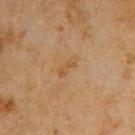biopsy status: no biopsy performed (imaged during a skin exam) | anatomic site: the back | lesion diameter: ~3 mm (longest diameter) | tile lighting: cross-polarized | image: total-body-photography crop, ~15 mm field of view | patient: male, aged 43–47.